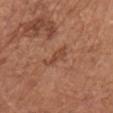notes = no biopsy performed (imaged during a skin exam)
anatomic site = the front of the torso
diameter = about 3 mm
patient = female, aged 73 to 77
lighting = white-light
acquisition = total-body-photography crop, ~15 mm field of view